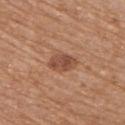biopsy status: no biopsy performed (imaged during a skin exam).